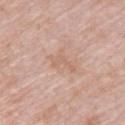Impression:
The lesion was photographed on a routine skin check and not biopsied; there is no pathology result.
Acquisition and patient details:
A female subject, approximately 65 years of age. Imaged with white-light lighting. From the upper back. A 15 mm crop from a total-body photograph taken for skin-cancer surveillance. Approximately 3.5 mm at its widest. Automated tile analysis of the lesion measured roughly 6 lightness units darker than nearby skin and a lesion-to-skin contrast of about 4.5 (normalized; higher = more distinct). The analysis additionally found an automated nevus-likeness rating near 0 out of 100 and a lesion-detection confidence of about 100/100.